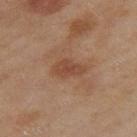tile lighting: cross-polarized; patient: female, aged approximately 60; image source: ~15 mm crop, total-body skin-cancer survey; size: ≈4 mm; site: the leg.